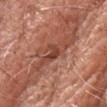biopsy status = imaged on a skin check; not biopsied
diameter = about 2.5 mm
automated lesion analysis = roughly 11 lightness units darker than nearby skin and a normalized lesion–skin contrast near 8
tile lighting = white-light illumination
site = the head or neck
imaging modality = 15 mm crop, total-body photography
subject = male, aged 78–82